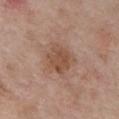– follow-up · imaged on a skin check; not biopsied
– patient · female, aged 83 or over
– image-analysis metrics · a lesion-to-skin contrast of about 6.5 (normalized; higher = more distinct); a border-irregularity rating of about 2.5/10, internal color variation of about 3 on a 0–10 scale, and peripheral color asymmetry of about 1; a lesion-detection confidence of about 100/100
– illumination · white-light illumination
– image source · total-body-photography crop, ~15 mm field of view
– body site · the chest